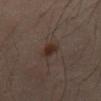Captured during whole-body skin photography for melanoma surveillance; the lesion was not biopsied. The tile uses cross-polarized illumination. On the abdomen. The total-body-photography lesion software estimated a mean CIELAB color near L≈28 a*≈15 b*≈21, a lesion–skin lightness drop of about 8, and a lesion-to-skin contrast of about 9 (normalized; higher = more distinct). The analysis additionally found border irregularity of about 2 on a 0–10 scale. The subject is a male aged around 65. About 2.5 mm across. This image is a 15 mm lesion crop taken from a total-body photograph.